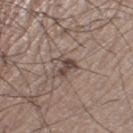{"biopsy_status": "not biopsied; imaged during a skin examination", "lighting": "white-light", "site": "left thigh", "image": {"source": "total-body photography crop", "field_of_view_mm": 15}, "automated_metrics": {"area_mm2_approx": 4.0, "shape_asymmetry": 0.6, "color_variation_0_10": 1.5, "peripheral_color_asymmetry": 1.0}, "patient": {"sex": "male", "age_approx": 60}, "lesion_size": {"long_diameter_mm_approx": 3.0}}